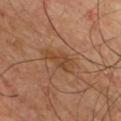<lesion>
<biopsy_status>not biopsied; imaged during a skin examination</biopsy_status>
<site>front of the torso</site>
<lighting>cross-polarized</lighting>
<image>
  <source>total-body photography crop</source>
  <field_of_view_mm>15</field_of_view_mm>
</image>
<patient>
  <sex>male</sex>
  <age_approx>40</age_approx>
</patient>
<lesion_size>
  <long_diameter_mm_approx>4.5</long_diameter_mm_approx>
</lesion_size>
</lesion>